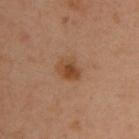Part of a total-body skin-imaging series; this lesion was reviewed on a skin check and was not flagged for biopsy. Located on the right upper arm. Automated tile analysis of the lesion measured a border-irregularity rating of about 2/10, a color-variation rating of about 4/10, and peripheral color asymmetry of about 1. This image is a 15 mm lesion crop taken from a total-body photograph. A male patient, aged 38–42.